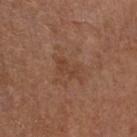Assessment:
Imaged during a routine full-body skin examination; the lesion was not biopsied and no histopathology is available.
Image and clinical context:
A male subject, aged around 70. Measured at roughly 3.5 mm in maximum diameter. Automated tile analysis of the lesion measured a footprint of about 4.5 mm², an eccentricity of roughly 0.85, and two-axis asymmetry of about 0.45. It also reported border irregularity of about 6 on a 0–10 scale, a within-lesion color-variation index near 1/10, and radial color variation of about 0. It also reported a lesion-detection confidence of about 100/100. Captured under white-light illumination. The lesion is on the chest. Cropped from a total-body skin-imaging series; the visible field is about 15 mm.